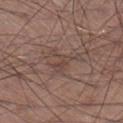Impression: This lesion was catalogued during total-body skin photography and was not selected for biopsy. Context: A male subject, about 55 years old. Located on the leg. Cropped from a whole-body photographic skin survey; the tile spans about 15 mm. The tile uses white-light illumination.